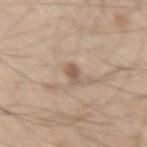Acquisition and patient details:
This is a white-light tile. The total-body-photography lesion software estimated an average lesion color of about L≈56 a*≈15 b*≈27 (CIELAB), roughly 10 lightness units darker than nearby skin, and a normalized lesion–skin contrast near 7. The analysis additionally found border irregularity of about 2.5 on a 0–10 scale, internal color variation of about 1.5 on a 0–10 scale, and a peripheral color-asymmetry measure near 0. And it measured a classifier nevus-likeness of about 25/100 and a lesion-detection confidence of about 100/100. The lesion is located on the right thigh. Approximately 2.5 mm at its widest. A male patient roughly 60 years of age. A close-up tile cropped from a whole-body skin photograph, about 15 mm across.